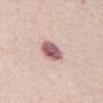– workup · total-body-photography surveillance lesion; no biopsy